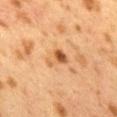  biopsy_status: not biopsied; imaged during a skin examination
  site: mid back
  patient:
    sex: female
    age_approx: 40
  lighting: cross-polarized
  image:
    source: total-body photography crop
    field_of_view_mm: 15
  lesion_size:
    long_diameter_mm_approx: 3.0